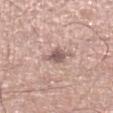<lesion>
  <biopsy_status>not biopsied; imaged during a skin examination</biopsy_status>
  <patient>
    <sex>male</sex>
    <age_approx>55</age_approx>
  </patient>
  <lighting>white-light</lighting>
  <site>left lower leg</site>
  <image>
    <source>total-body photography crop</source>
    <field_of_view_mm>15</field_of_view_mm>
  </image>
</lesion>